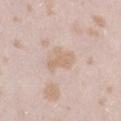tile lighting: white-light illumination
lesion diameter: about 4 mm
automated metrics: an average lesion color of about L≈67 a*≈15 b*≈29 (CIELAB) and a normalized lesion–skin contrast near 6
image source: 15 mm crop, total-body photography
subject: female, aged around 25
body site: the right thigh